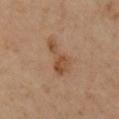This lesion was catalogued during total-body skin photography and was not selected for biopsy.
A male patient, aged approximately 65.
The recorded lesion diameter is about 5 mm.
An algorithmic analysis of the crop reported a lesion color around L≈49 a*≈20 b*≈32 in CIELAB, a lesion–skin lightness drop of about 9, and a normalized lesion–skin contrast near 7. It also reported an automated nevus-likeness rating near 20 out of 100 and a lesion-detection confidence of about 100/100.
From the right lower leg.
A 15 mm close-up tile from a total-body photography series done for melanoma screening.
The tile uses cross-polarized illumination.